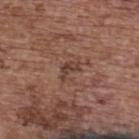biopsy_status: not biopsied; imaged during a skin examination
site: upper back
patient:
  sex: female
  age_approx: 65
automated_metrics:
  border_irregularity_0_10: 5.5
  color_variation_0_10: 0.0
  peripheral_color_asymmetry: 0.0
  nevus_likeness_0_100: 0
  lesion_detection_confidence_0_100: 100
lesion_size:
  long_diameter_mm_approx: 2.5
image:
  source: total-body photography crop
  field_of_view_mm: 15
lighting: white-light